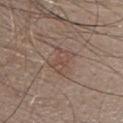biopsy status = catalogued during a skin exam; not biopsied
patient = male, roughly 50 years of age
lesion size = about 3.5 mm
image-analysis metrics = two-axis asymmetry of about 0.35
image = 15 mm crop, total-body photography
tile lighting = white-light illumination
body site = the back